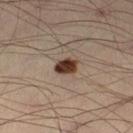follow-up: total-body-photography surveillance lesion; no biopsy | patient: male, aged 38–42 | imaging modality: 15 mm crop, total-body photography | lighting: cross-polarized illumination | body site: the left lower leg | lesion diameter: ~3 mm (longest diameter).